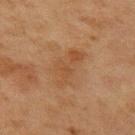Imaged during a routine full-body skin examination; the lesion was not biopsied and no histopathology is available.
Longest diameter approximately 5.5 mm.
From the left upper arm.
Imaged with cross-polarized lighting.
This image is a 15 mm lesion crop taken from a total-body photograph.
A female subject aged around 40.